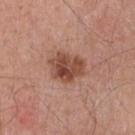workup — total-body-photography surveillance lesion; no biopsy